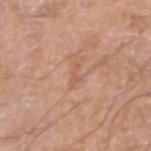<record>
<biopsy_status>not biopsied; imaged during a skin examination</biopsy_status>
<site>right upper arm</site>
<lighting>white-light</lighting>
<automated_metrics>
  <cielab_L>58</cielab_L>
  <cielab_a>22</cielab_a>
  <cielab_b>31</cielab_b>
  <vs_skin_contrast_norm>4.5</vs_skin_contrast_norm>
  <color_variation_0_10>0.0</color_variation_0_10>
  <peripheral_color_asymmetry>0.0</peripheral_color_asymmetry>
  <nevus_likeness_0_100>0</nevus_likeness_0_100>
  <lesion_detection_confidence_0_100>95</lesion_detection_confidence_0_100>
</automated_metrics>
<lesion_size>
  <long_diameter_mm_approx>2.5</long_diameter_mm_approx>
</lesion_size>
<patient>
  <sex>male</sex>
  <age_approx>55</age_approx>
</patient>
<image>
  <source>total-body photography crop</source>
  <field_of_view_mm>15</field_of_view_mm>
</image>
</record>